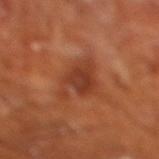| feature | finding |
|---|---|
| notes | total-body-photography surveillance lesion; no biopsy |
| site | the right lower leg |
| acquisition | ~15 mm tile from a whole-body skin photo |
| subject | male, aged 63 to 67 |
| TBP lesion metrics | an area of roughly 10 mm², a shape eccentricity near 0.85, and a shape-asymmetry score of about 0.35 (0 = symmetric); border irregularity of about 5.5 on a 0–10 scale, a within-lesion color-variation index near 3/10, and peripheral color asymmetry of about 1 |
| tile lighting | cross-polarized |